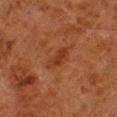biopsy status = no biopsy performed (imaged during a skin exam); body site = the leg; acquisition = total-body-photography crop, ~15 mm field of view; automated metrics = an average lesion color of about L≈29 a*≈22 b*≈29 (CIELAB), about 6 CIELAB-L* units darker than the surrounding skin, and a normalized lesion–skin contrast near 6; diameter = about 3.5 mm; patient = male, roughly 80 years of age.